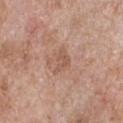Assessment: Imaged during a routine full-body skin examination; the lesion was not biopsied and no histopathology is available. Context: The tile uses white-light illumination. Measured at roughly 3 mm in maximum diameter. A roughly 15 mm field-of-view crop from a total-body skin photograph. A male subject aged around 60. The lesion-visualizer software estimated a mean CIELAB color near L≈55 a*≈20 b*≈29, roughly 7 lightness units darker than nearby skin, and a normalized border contrast of about 5.5. And it measured a border-irregularity rating of about 5/10, a within-lesion color-variation index near 1.5/10, and a peripheral color-asymmetry measure near 0.5. The analysis additionally found a classifier nevus-likeness of about 0/100 and a detector confidence of about 100 out of 100 that the crop contains a lesion. Located on the chest.